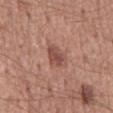notes: total-body-photography surveillance lesion; no biopsy
imaging modality: 15 mm crop, total-body photography
anatomic site: the back
patient: male, in their mid-50s
illumination: white-light illumination
image-analysis metrics: an area of roughly 6 mm², an outline eccentricity of about 0.75 (0 = round, 1 = elongated), and a shape-asymmetry score of about 0.25 (0 = symmetric)